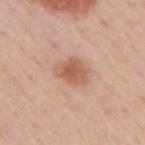Imaged during a routine full-body skin examination; the lesion was not biopsied and no histopathology is available. The lesion is located on the right upper arm. Automated tile analysis of the lesion measured an outline eccentricity of about 0.7 (0 = round, 1 = elongated) and a symmetry-axis asymmetry near 0.25. And it measured a mean CIELAB color near L≈59 a*≈23 b*≈32, about 11 CIELAB-L* units darker than the surrounding skin, and a normalized lesion–skin contrast near 7.5. The analysis additionally found a border-irregularity index near 2.5/10, internal color variation of about 3 on a 0–10 scale, and radial color variation of about 1. It also reported a classifier nevus-likeness of about 85/100. Cropped from a whole-body photographic skin survey; the tile spans about 15 mm. A male patient aged around 60. Longest diameter approximately 4 mm.